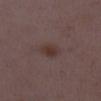{
  "biopsy_status": "not biopsied; imaged during a skin examination",
  "image": {
    "source": "total-body photography crop",
    "field_of_view_mm": 15
  },
  "lesion_size": {
    "long_diameter_mm_approx": 2.5
  },
  "site": "right lower leg",
  "automated_metrics": {
    "area_mm2_approx": 5.0,
    "shape_asymmetry": 0.15,
    "cielab_L": 34,
    "cielab_a": 16,
    "cielab_b": 19,
    "color_variation_0_10": 2.0,
    "peripheral_color_asymmetry": 0.5
  },
  "lighting": "white-light",
  "patient": {
    "sex": "female",
    "age_approx": 30
  }
}